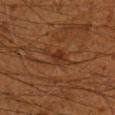follow-up = no biopsy performed (imaged during a skin exam)
lighting = cross-polarized illumination
site = the right lower leg
size = ~4 mm (longest diameter)
subject = male, aged 58 to 62
acquisition = 15 mm crop, total-body photography